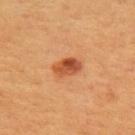image — total-body-photography crop, ~15 mm field of view; patient — male, aged approximately 55; illumination — cross-polarized illumination; body site — the mid back; lesion diameter — about 3.5 mm.